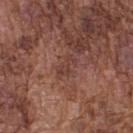Assessment: This lesion was catalogued during total-body skin photography and was not selected for biopsy. Image and clinical context: This is a white-light tile. A close-up tile cropped from a whole-body skin photograph, about 15 mm across. Automated image analysis of the tile measured a lesion area of about 3.5 mm² and a shape-asymmetry score of about 0.45 (0 = symmetric). The analysis additionally found an average lesion color of about L≈40 a*≈22 b*≈24 (CIELAB), roughly 7 lightness units darker than nearby skin, and a normalized lesion–skin contrast near 5.5. The software also gave a border-irregularity rating of about 5.5/10, internal color variation of about 1 on a 0–10 scale, and a peripheral color-asymmetry measure near 0.5. The subject is a male about 75 years old. The recorded lesion diameter is about 3 mm. The lesion is on the upper back.